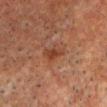Q: Was a biopsy performed?
A: catalogued during a skin exam; not biopsied
Q: What are the patient's age and sex?
A: male, aged approximately 60
Q: What is the anatomic site?
A: the head or neck
Q: What kind of image is this?
A: ~15 mm tile from a whole-body skin photo